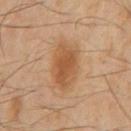Impression:
Recorded during total-body skin imaging; not selected for excision or biopsy.
Context:
A region of skin cropped from a whole-body photographic capture, roughly 15 mm wide. On the chest. A male patient aged 58 to 62.